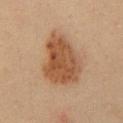Assessment:
No biopsy was performed on this lesion — it was imaged during a full skin examination and was not determined to be concerning.
Clinical summary:
On the front of the torso. Captured under cross-polarized illumination. The patient is a male approximately 35 years of age. Cropped from a whole-body photographic skin survey; the tile spans about 15 mm. Measured at roughly 7 mm in maximum diameter.